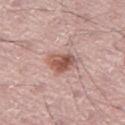patient — male, aged 68–72
TBP lesion metrics — roughly 13 lightness units darker than nearby skin and a lesion-to-skin contrast of about 8.5 (normalized; higher = more distinct)
anatomic site — the leg
image — total-body-photography crop, ~15 mm field of view